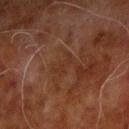No biopsy was performed on this lesion — it was imaged during a full skin examination and was not determined to be concerning.